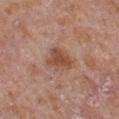{
  "biopsy_status": "not biopsied; imaged during a skin examination",
  "image": {
    "source": "total-body photography crop",
    "field_of_view_mm": 15
  },
  "lighting": "white-light",
  "patient": {
    "sex": "male",
    "age_approx": 65
  },
  "automated_metrics": {
    "area_mm2_approx": 7.5,
    "shape_asymmetry": 0.35,
    "border_irregularity_0_10": 3.0,
    "color_variation_0_10": 3.0,
    "peripheral_color_asymmetry": 1.0,
    "lesion_detection_confidence_0_100": 100
  },
  "site": "chest"
}